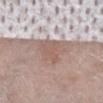Findings:
- notes · total-body-photography surveillance lesion; no biopsy
- lesion diameter · about 6.5 mm
- acquisition · ~15 mm crop, total-body skin-cancer survey
- body site · the leg
- subject · male, aged around 60
- lighting · white-light